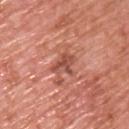follow-up: imaged on a skin check; not biopsied | image-analysis metrics: an area of roughly 5.5 mm² and a shape eccentricity near 0.75; a lesion color around L≈51 a*≈28 b*≈30 in CIELAB, a lesion–skin lightness drop of about 10, and a normalized border contrast of about 7; a color-variation rating of about 3/10 and peripheral color asymmetry of about 1 | location: the back | patient: male, in their 70s | diameter: about 3.5 mm | acquisition: ~15 mm crop, total-body skin-cancer survey | lighting: white-light illumination.